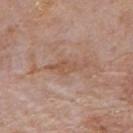A region of skin cropped from a whole-body photographic capture, roughly 15 mm wide. From the upper back. The lesion's longest dimension is about 4.5 mm. A male patient aged 73–77.